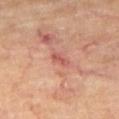Image and clinical context: A patient in their mid-60s. About 2.5 mm across. The tile uses cross-polarized illumination. Cropped from a whole-body photographic skin survey; the tile spans about 15 mm. The lesion is on the right thigh.